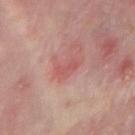{"biopsy_status": "not biopsied; imaged during a skin examination", "image": {"source": "total-body photography crop", "field_of_view_mm": 15}, "site": "right forearm", "lighting": "white-light", "lesion_size": {"long_diameter_mm_approx": 3.0}, "patient": {"sex": "male", "age_approx": 75}}